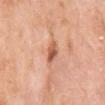The lesion was tiled from a total-body skin photograph and was not biopsied. On the left upper arm. The tile uses white-light illumination. Longest diameter approximately 3 mm. Cropped from a whole-body photographic skin survey; the tile spans about 15 mm. A female patient, aged around 70.